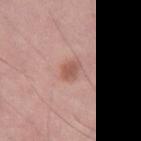The lesion was photographed on a routine skin check and not biopsied; there is no pathology result. The tile uses white-light illumination. This image is a 15 mm lesion crop taken from a total-body photograph. The lesion is on the right thigh. The recorded lesion diameter is about 2.5 mm. A female patient, about 40 years old. The total-body-photography lesion software estimated internal color variation of about 1.5 on a 0–10 scale and a peripheral color-asymmetry measure near 0.5. The analysis additionally found a classifier nevus-likeness of about 65/100 and a lesion-detection confidence of about 100/100.